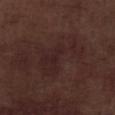| field | value |
|---|---|
| workup | catalogued during a skin exam; not biopsied |